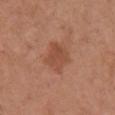Impression: The lesion was photographed on a routine skin check and not biopsied; there is no pathology result. Context: A female patient aged 63–67. A close-up tile cropped from a whole-body skin photograph, about 15 mm across. On the chest.